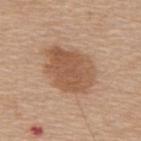Clinical impression:
This lesion was catalogued during total-body skin photography and was not selected for biopsy.
Acquisition and patient details:
Longest diameter approximately 6.5 mm. A male patient, aged around 65. On the upper back. The tile uses white-light illumination. Cropped from a total-body skin-imaging series; the visible field is about 15 mm.